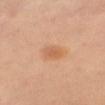The lesion was tiled from a total-body skin photograph and was not biopsied. A close-up tile cropped from a whole-body skin photograph, about 15 mm across. The subject is a female aged approximately 40. The total-body-photography lesion software estimated a mean CIELAB color near L≈62 a*≈24 b*≈38, about 8 CIELAB-L* units darker than the surrounding skin, and a normalized border contrast of about 5.5. Approximately 2.5 mm at its widest. The tile uses cross-polarized illumination. From the left leg.